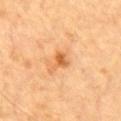Case summary:
• follow-up · no biopsy performed (imaged during a skin exam)
• lesion diameter · ~2.5 mm (longest diameter)
• image · 15 mm crop, total-body photography
• image-analysis metrics · an area of roughly 4 mm², a shape eccentricity near 0.7, and a symmetry-axis asymmetry near 0.45; a border-irregularity rating of about 4/10 and a color-variation rating of about 2.5/10; a classifier nevus-likeness of about 45/100 and a detector confidence of about 100 out of 100 that the crop contains a lesion
• site · the chest
• subject · male, roughly 85 years of age or older